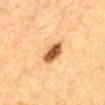Captured during whole-body skin photography for melanoma surveillance; the lesion was not biopsied. Located on the abdomen. Captured under cross-polarized illumination. The lesion-visualizer software estimated a lesion area of about 6 mm², an eccentricity of roughly 0.7, and a symmetry-axis asymmetry near 0.2. The analysis additionally found about 18 CIELAB-L* units darker than the surrounding skin and a lesion-to-skin contrast of about 12 (normalized; higher = more distinct). The analysis additionally found a border-irregularity rating of about 1.5/10, a color-variation rating of about 4.5/10, and radial color variation of about 2. The analysis additionally found a nevus-likeness score of about 100/100 and a lesion-detection confidence of about 100/100. The recorded lesion diameter is about 3 mm. A male patient about 85 years old or older. A 15 mm crop from a total-body photograph taken for skin-cancer surveillance.